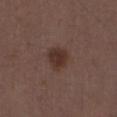Q: Was this lesion biopsied?
A: imaged on a skin check; not biopsied
Q: What kind of image is this?
A: total-body-photography crop, ~15 mm field of view
Q: Illumination type?
A: white-light
Q: Lesion size?
A: ~3 mm (longest diameter)
Q: Patient demographics?
A: male, approximately 30 years of age
Q: What is the anatomic site?
A: the abdomen